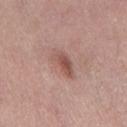<lesion>
  <biopsy_status>not biopsied; imaged during a skin examination</biopsy_status>
  <lesion_size>
    <long_diameter_mm_approx>4.0</long_diameter_mm_approx>
  </lesion_size>
  <patient>
    <sex>female</sex>
    <age_approx>40</age_approx>
  </patient>
  <image>
    <source>total-body photography crop</source>
    <field_of_view_mm>15</field_of_view_mm>
  </image>
  <lighting>white-light</lighting>
  <site>left thigh</site>
</lesion>